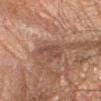– notes: catalogued during a skin exam; not biopsied